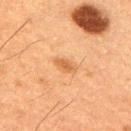biopsy_status: not biopsied; imaged during a skin examination
site: right upper arm
lesion_size:
  long_diameter_mm_approx: 2.5
automated_metrics:
  area_mm2_approx: 3.0
  eccentricity: 0.85
  shape_asymmetry: 0.3
  cielab_L: 48
  cielab_a: 21
  cielab_b: 36
  vs_skin_darker_L: 8.0
  vs_skin_contrast_norm: 6.5
  nevus_likeness_0_100: 10
lighting: cross-polarized
patient:
  sex: male
  age_approx: 50
image:
  source: total-body photography crop
  field_of_view_mm: 15